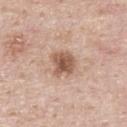biopsy status: catalogued during a skin exam; not biopsied | lighting: white-light | anatomic site: the upper back | automated metrics: a lesion area of about 7.5 mm²; an average lesion color of about L≈56 a*≈19 b*≈28 (CIELAB) and a lesion-to-skin contrast of about 9 (normalized; higher = more distinct); a classifier nevus-likeness of about 70/100 and a detector confidence of about 100 out of 100 that the crop contains a lesion | lesion diameter: ~3 mm (longest diameter) | subject: male, aged around 45 | acquisition: total-body-photography crop, ~15 mm field of view.